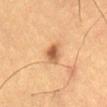{"image": {"source": "total-body photography crop", "field_of_view_mm": 15}, "site": "front of the torso", "patient": {"sex": "male", "age_approx": 55}}